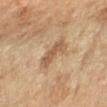notes = total-body-photography surveillance lesion; no biopsy | image source = ~15 mm tile from a whole-body skin photo | automated lesion analysis = a lesion area of about 7.5 mm² and a shape eccentricity near 0.85; a lesion color around L≈58 a*≈18 b*≈35 in CIELAB, roughly 10 lightness units darker than nearby skin, and a normalized border contrast of about 6.5; border irregularity of about 5 on a 0–10 scale, internal color variation of about 2 on a 0–10 scale, and radial color variation of about 0.5 | lighting = cross-polarized | lesion diameter = about 4 mm | patient = female, in their 60s | location = the arm.